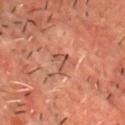The lesion was tiled from a total-body skin photograph and was not biopsied.
From the chest.
A 15 mm close-up extracted from a 3D total-body photography capture.
The patient is a male aged 48 to 52.